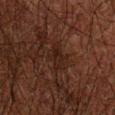Notes:
– biopsy status · catalogued during a skin exam; not biopsied
– image · 15 mm crop, total-body photography
– illumination · cross-polarized
– diameter · ≈3 mm
– location · the right forearm
– subject · male, approximately 50 years of age